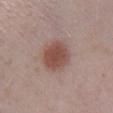Q: Was a biopsy performed?
A: imaged on a skin check; not biopsied